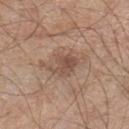Assessment:
The lesion was photographed on a routine skin check and not biopsied; there is no pathology result.
Context:
A 15 mm crop from a total-body photograph taken for skin-cancer surveillance. The subject is a male approximately 60 years of age. The lesion is on the left lower leg.